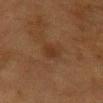follow-up=total-body-photography surveillance lesion; no biopsy
lesion diameter=≈2.5 mm
tile lighting=cross-polarized
body site=the chest
image=~15 mm tile from a whole-body skin photo
patient=female, in their mid-50s